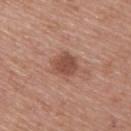Q: Was a biopsy performed?
A: total-body-photography surveillance lesion; no biopsy
Q: What are the patient's age and sex?
A: female, aged around 60
Q: What lighting was used for the tile?
A: white-light illumination
Q: Where on the body is the lesion?
A: the upper back
Q: What kind of image is this?
A: total-body-photography crop, ~15 mm field of view
Q: What is the lesion's diameter?
A: about 3.5 mm